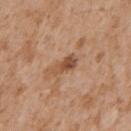Findings:
• follow-up — total-body-photography surveillance lesion; no biopsy
• body site — the chest
• subject — male, roughly 65 years of age
• lesion diameter — about 3.5 mm
• imaging modality — 15 mm crop, total-body photography
• image-analysis metrics — a lesion color around L≈51 a*≈21 b*≈32 in CIELAB and a lesion-to-skin contrast of about 8 (normalized; higher = more distinct)